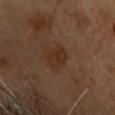Captured during whole-body skin photography for melanoma surveillance; the lesion was not biopsied. This image is a 15 mm lesion crop taken from a total-body photograph. Captured under cross-polarized illumination. Longest diameter approximately 3.5 mm. Automated image analysis of the tile measured an area of roughly 5.5 mm², an outline eccentricity of about 0.8 (0 = round, 1 = elongated), and a symmetry-axis asymmetry near 0.25. And it measured a mean CIELAB color near L≈25 a*≈16 b*≈24, roughly 5 lightness units darker than nearby skin, and a normalized lesion–skin contrast near 6.5. And it measured a border-irregularity index near 2.5/10, internal color variation of about 2.5 on a 0–10 scale, and peripheral color asymmetry of about 1. And it measured a classifier nevus-likeness of about 15/100. The lesion is on the head or neck. A female patient, aged 58 to 62.